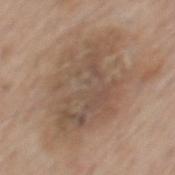The lesion was tiled from a total-body skin photograph and was not biopsied. The lesion-visualizer software estimated a lesion color around L≈52 a*≈15 b*≈27 in CIELAB and a normalized lesion–skin contrast near 6.5. The software also gave a border-irregularity index near 7/10 and radial color variation of about 1.5. A male subject, approximately 55 years of age. From the mid back. Cropped from a whole-body photographic skin survey; the tile spans about 15 mm.